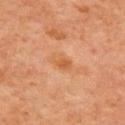biopsy status — imaged on a skin check; not biopsied